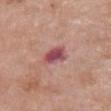The lesion is on the chest.
This image is a 15 mm lesion crop taken from a total-body photograph.
A female patient, in their mid-70s.
Automated tile analysis of the lesion measured a footprint of about 6.5 mm², an outline eccentricity of about 0.7 (0 = round, 1 = elongated), and a shape-asymmetry score of about 0.25 (0 = symmetric). And it measured a border-irregularity rating of about 2.5/10, a within-lesion color-variation index near 4.5/10, and a peripheral color-asymmetry measure near 1.5.
Measured at roughly 3.5 mm in maximum diameter.
Imaged with white-light lighting.